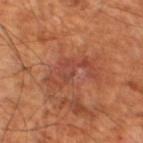Recorded during total-body skin imaging; not selected for excision or biopsy.
Imaged with cross-polarized lighting.
Automated tile analysis of the lesion measured a border-irregularity rating of about 8/10, a within-lesion color-variation index near 3/10, and a peripheral color-asymmetry measure near 0.5.
The lesion is located on the left lower leg.
A 15 mm crop from a total-body photograph taken for skin-cancer surveillance.
The recorded lesion diameter is about 6 mm.
A male patient, roughly 60 years of age.